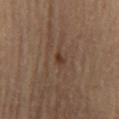Part of a total-body skin-imaging series; this lesion was reviewed on a skin check and was not flagged for biopsy. Longest diameter approximately 2 mm. A roughly 15 mm field-of-view crop from a total-body skin photograph. A female patient, roughly 65 years of age. The lesion is located on the back.